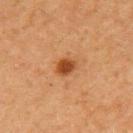follow-up: catalogued during a skin exam; not biopsied
size: ~2.5 mm (longest diameter)
location: the left upper arm
automated metrics: border irregularity of about 1.5 on a 0–10 scale, internal color variation of about 3.5 on a 0–10 scale, and peripheral color asymmetry of about 1.5
tile lighting: cross-polarized illumination
imaging modality: ~15 mm crop, total-body skin-cancer survey
patient: female, aged 58–62